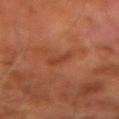<tbp_lesion>
  <biopsy_status>not biopsied; imaged during a skin examination</biopsy_status>
  <automated_metrics>
    <area_mm2_approx>3.5</area_mm2_approx>
    <shape_asymmetry>0.35</shape_asymmetry>
    <cielab_L>39</cielab_L>
    <cielab_a>26</cielab_a>
    <cielab_b>32</cielab_b>
    <vs_skin_darker_L>6.0</vs_skin_darker_L>
  </automated_metrics>
  <image>
    <source>total-body photography crop</source>
    <field_of_view_mm>15</field_of_view_mm>
  </image>
  <site>left forearm</site>
  <lighting>cross-polarized</lighting>
  <patient>
    <sex>male</sex>
    <age_approx>60</age_approx>
  </patient>
</tbp_lesion>